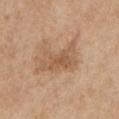Q: Was a biopsy performed?
A: no biopsy performed (imaged during a skin exam)
Q: What are the patient's age and sex?
A: male, aged 63 to 67
Q: What did automated image analysis measure?
A: an outline eccentricity of about 0.85 (0 = round, 1 = elongated); a lesion color around L≈56 a*≈18 b*≈34 in CIELAB, a lesion–skin lightness drop of about 9, and a normalized border contrast of about 6; border irregularity of about 5.5 on a 0–10 scale and radial color variation of about 1
Q: How was this image acquired?
A: ~15 mm crop, total-body skin-cancer survey
Q: Lesion location?
A: the chest
Q: Lesion size?
A: ~5 mm (longest diameter)